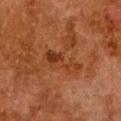Impression:
The lesion was tiled from a total-body skin photograph and was not biopsied.
Acquisition and patient details:
A female subject, aged 48–52. On the chest. Imaged with cross-polarized lighting. A 15 mm close-up extracted from a 3D total-body photography capture.